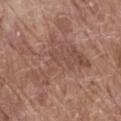Assessment:
The lesion was tiled from a total-body skin photograph and was not biopsied.
Image and clinical context:
Automated tile analysis of the lesion measured a mean CIELAB color near L≈49 a*≈20 b*≈25, roughly 7 lightness units darker than nearby skin, and a normalized lesion–skin contrast near 5.5. This image is a 15 mm lesion crop taken from a total-body photograph. The patient is a male about 80 years old. Imaged with white-light lighting. From the abdomen. Measured at roughly 8.5 mm in maximum diameter.